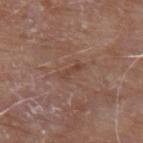biopsy status = imaged on a skin check; not biopsied
illumination = white-light illumination
TBP lesion metrics = an area of roughly 2.5 mm², a shape eccentricity near 0.95, and a symmetry-axis asymmetry near 0.45; a lesion–skin lightness drop of about 6 and a normalized border contrast of about 5.5; a nevus-likeness score of about 0/100 and a lesion-detection confidence of about 100/100
location = the arm
patient = male, in their 80s
image source = ~15 mm crop, total-body skin-cancer survey
lesion size = ≈3 mm